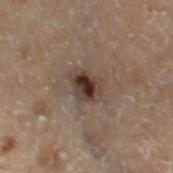workup — no biopsy performed (imaged during a skin exam)
anatomic site — the left thigh
tile lighting — cross-polarized illumination
subject — male, aged around 70
acquisition — 15 mm crop, total-body photography
automated metrics — a detector confidence of about 100 out of 100 that the crop contains a lesion